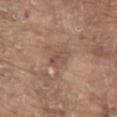Captured during whole-body skin photography for melanoma surveillance; the lesion was not biopsied.
From the abdomen.
The total-body-photography lesion software estimated a border-irregularity rating of about 3.5/10, a within-lesion color-variation index near 1.5/10, and a peripheral color-asymmetry measure near 0.5. The software also gave a nevus-likeness score of about 0/100 and lesion-presence confidence of about 90/100.
Cropped from a whole-body photographic skin survey; the tile spans about 15 mm.
The tile uses white-light illumination.
The subject is a male aged around 80.